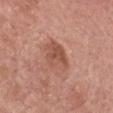Notes:
* notes · imaged on a skin check; not biopsied
* TBP lesion metrics · a footprint of about 9.5 mm² and a symmetry-axis asymmetry near 0.15; internal color variation of about 4.5 on a 0–10 scale and radial color variation of about 1.5
* patient · male, aged around 75
* location · the head or neck
* tile lighting · white-light illumination
* image · 15 mm crop, total-body photography
* size · ~4 mm (longest diameter)